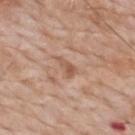Findings:
– follow-up — catalogued during a skin exam; not biopsied
– imaging modality — total-body-photography crop, ~15 mm field of view
– subject — male, aged approximately 60
– illumination — white-light
– diameter — about 3 mm
– site — the back
– automated lesion analysis — a footprint of about 3.5 mm² and a shape eccentricity near 0.9; an average lesion color of about L≈56 a*≈20 b*≈30 (CIELAB), about 9 CIELAB-L* units darker than the surrounding skin, and a normalized border contrast of about 6; a border-irregularity index near 4.5/10, internal color variation of about 1 on a 0–10 scale, and a peripheral color-asymmetry measure near 0.5; a classifier nevus-likeness of about 0/100 and a lesion-detection confidence of about 100/100